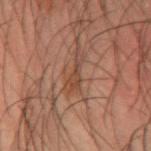Clinical impression: Part of a total-body skin-imaging series; this lesion was reviewed on a skin check and was not flagged for biopsy. Image and clinical context: The subject is a male in their 50s. Measured at roughly 4 mm in maximum diameter. On the left forearm. The tile uses cross-polarized illumination. A 15 mm crop from a total-body photograph taken for skin-cancer surveillance.